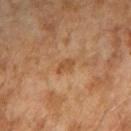biopsy status = imaged on a skin check; not biopsied
subject = female, approximately 60 years of age
location = the right upper arm
image source = 15 mm crop, total-body photography
lesion size = about 2.5 mm
lighting = cross-polarized
automated lesion analysis = border irregularity of about 2.5 on a 0–10 scale and a color-variation rating of about 1/10; an automated nevus-likeness rating near 5 out of 100